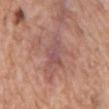Findings:
– follow-up — catalogued during a skin exam; not biopsied
– acquisition — 15 mm crop, total-body photography
– lighting — white-light
– site — the abdomen
– patient — male, aged 58–62